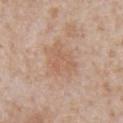Impression: Part of a total-body skin-imaging series; this lesion was reviewed on a skin check and was not flagged for biopsy. Clinical summary: The tile uses white-light illumination. The recorded lesion diameter is about 5 mm. A roughly 15 mm field-of-view crop from a total-body skin photograph. The lesion is on the front of the torso. An algorithmic analysis of the crop reported a lesion area of about 15 mm² and an outline eccentricity of about 0.55 (0 = round, 1 = elongated). The analysis additionally found a lesion-to-skin contrast of about 5 (normalized; higher = more distinct). It also reported a border-irregularity index near 3.5/10 and a color-variation rating of about 2.5/10. A male subject in their mid-60s.